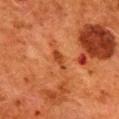Captured during whole-body skin photography for melanoma surveillance; the lesion was not biopsied. A female patient aged approximately 50. On the upper back. A lesion tile, about 15 mm wide, cut from a 3D total-body photograph. Approximately 2.5 mm at its widest. The lesion-visualizer software estimated an area of roughly 2.5 mm² and an outline eccentricity of about 0.9 (0 = round, 1 = elongated). The software also gave a lesion color around L≈38 a*≈28 b*≈36 in CIELAB and a lesion-to-skin contrast of about 7.5 (normalized; higher = more distinct). And it measured a border-irregularity index near 3/10, internal color variation of about 0.5 on a 0–10 scale, and radial color variation of about 0. The analysis additionally found a detector confidence of about 100 out of 100 that the crop contains a lesion.